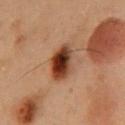illumination = cross-polarized illumination; site = the chest; patient = male, roughly 55 years of age; acquisition = ~15 mm tile from a whole-body skin photo.